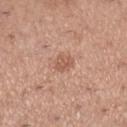Clinical impression:
No biopsy was performed on this lesion — it was imaged during a full skin examination and was not determined to be concerning.
Acquisition and patient details:
A 15 mm crop from a total-body photograph taken for skin-cancer surveillance. Longest diameter approximately 2.5 mm. The lesion-visualizer software estimated a footprint of about 3.5 mm², an outline eccentricity of about 0.7 (0 = round, 1 = elongated), and a shape-asymmetry score of about 0.3 (0 = symmetric). And it measured a normalized lesion–skin contrast near 6. The analysis additionally found border irregularity of about 3 on a 0–10 scale, a color-variation rating of about 1.5/10, and peripheral color asymmetry of about 0.5. The analysis additionally found an automated nevus-likeness rating near 15 out of 100 and a lesion-detection confidence of about 100/100. Imaged with white-light lighting. From the right lower leg. A female patient, about 30 years old.